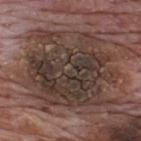biopsy status — total-body-photography surveillance lesion; no biopsy.